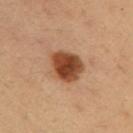Impression: Part of a total-body skin-imaging series; this lesion was reviewed on a skin check and was not flagged for biopsy. Background: The lesion is located on the right upper arm. About 4.5 mm across. A female patient approximately 35 years of age. A roughly 15 mm field-of-view crop from a total-body skin photograph. The lesion-visualizer software estimated an outline eccentricity of about 0.6 (0 = round, 1 = elongated) and two-axis asymmetry of about 0.2. And it measured an automated nevus-likeness rating near 100 out of 100 and a detector confidence of about 100 out of 100 that the crop contains a lesion. Captured under cross-polarized illumination.